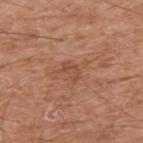Captured during whole-body skin photography for melanoma surveillance; the lesion was not biopsied.
The subject is a male in their mid-60s.
Imaged with white-light lighting.
This image is a 15 mm lesion crop taken from a total-body photograph.
Measured at roughly 2.5 mm in maximum diameter.
Located on the right upper arm.